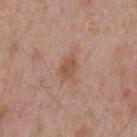Clinical impression:
Part of a total-body skin-imaging series; this lesion was reviewed on a skin check and was not flagged for biopsy.
Clinical summary:
From the mid back. This is a white-light tile. A roughly 15 mm field-of-view crop from a total-body skin photograph. The lesion-visualizer software estimated an area of roughly 4.5 mm², an eccentricity of roughly 0.8, and two-axis asymmetry of about 0.15. The analysis additionally found a lesion color around L≈53 a*≈21 b*≈30 in CIELAB, about 8 CIELAB-L* units darker than the surrounding skin, and a lesion-to-skin contrast of about 6.5 (normalized; higher = more distinct). And it measured border irregularity of about 2 on a 0–10 scale, internal color variation of about 1.5 on a 0–10 scale, and a peripheral color-asymmetry measure near 0.5. Measured at roughly 3.5 mm in maximum diameter. The patient is a male aged 53 to 57.